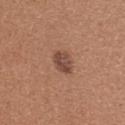Clinical impression: Imaged during a routine full-body skin examination; the lesion was not biopsied and no histopathology is available. Context: A female patient, roughly 55 years of age. Automated tile analysis of the lesion measured an area of roughly 5 mm², a shape eccentricity near 0.65, and two-axis asymmetry of about 0.2. And it measured border irregularity of about 2 on a 0–10 scale, a within-lesion color-variation index near 3.5/10, and peripheral color asymmetry of about 1. On the upper back. Cropped from a whole-body photographic skin survey; the tile spans about 15 mm.